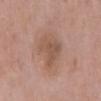workup: total-body-photography surveillance lesion; no biopsy | TBP lesion metrics: an average lesion color of about L≈53 a*≈19 b*≈27 (CIELAB), about 8 CIELAB-L* units darker than the surrounding skin, and a normalized lesion–skin contrast near 5.5; a border-irregularity rating of about 5/10, internal color variation of about 2 on a 0–10 scale, and a peripheral color-asymmetry measure near 0.5; a detector confidence of about 100 out of 100 that the crop contains a lesion | tile lighting: white-light illumination | subject: male, aged approximately 60 | diameter: ≈3.5 mm | site: the mid back | image source: total-body-photography crop, ~15 mm field of view.